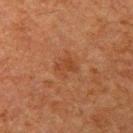The lesion was photographed on a routine skin check and not biopsied; there is no pathology result. This is a cross-polarized tile. A close-up tile cropped from a whole-body skin photograph, about 15 mm across. The recorded lesion diameter is about 2.5 mm. The total-body-photography lesion software estimated a mean CIELAB color near L≈33 a*≈21 b*≈29 and a lesion-to-skin contrast of about 5.5 (normalized; higher = more distinct). It also reported a border-irregularity index near 4/10, a color-variation rating of about 1.5/10, and radial color variation of about 0.5. The patient is a male aged 58–62. On the left upper arm.